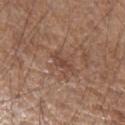Clinical impression:
The lesion was tiled from a total-body skin photograph and was not biopsied.
Image and clinical context:
The tile uses white-light illumination. Automated image analysis of the tile measured an area of roughly 3.5 mm², an outline eccentricity of about 0.85 (0 = round, 1 = elongated), and a shape-asymmetry score of about 0.4 (0 = symmetric). The analysis additionally found a within-lesion color-variation index near 1/10. A 15 mm close-up extracted from a 3D total-body photography capture. The subject is a male roughly 55 years of age. From the left forearm. The recorded lesion diameter is about 3 mm.